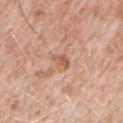Assessment:
The lesion was tiled from a total-body skin photograph and was not biopsied.
Clinical summary:
Captured under white-light illumination. On the mid back. The lesion-visualizer software estimated a lesion area of about 3 mm², a shape eccentricity near 0.85, and a shape-asymmetry score of about 0.35 (0 = symmetric). It also reported a lesion color around L≈58 a*≈22 b*≈32 in CIELAB and a lesion–skin lightness drop of about 9. The software also gave a detector confidence of about 100 out of 100 that the crop contains a lesion. A male patient, about 50 years old. A 15 mm crop from a total-body photograph taken for skin-cancer surveillance.